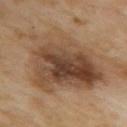workup=catalogued during a skin exam; not biopsied | lighting=cross-polarized | imaging modality=total-body-photography crop, ~15 mm field of view | body site=the upper back | patient=female, roughly 50 years of age | lesion size=about 10.5 mm.